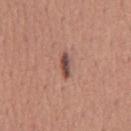biopsy status = imaged on a skin check; not biopsied | location = the chest | image source = ~15 mm tile from a whole-body skin photo | subject = male, in their mid-40s | lighting = white-light illumination | lesion diameter = about 3 mm.